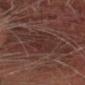Q: Is there a histopathology result?
A: total-body-photography surveillance lesion; no biopsy
Q: Automated lesion metrics?
A: a footprint of about 13 mm², a shape eccentricity near 0.65, and a symmetry-axis asymmetry near 0.3; an average lesion color of about L≈25 a*≈17 b*≈18 (CIELAB) and a normalized border contrast of about 5
Q: How was this image acquired?
A: ~15 mm tile from a whole-body skin photo
Q: What is the lesion's diameter?
A: about 5 mm
Q: Patient demographics?
A: male, aged 63 to 67
Q: Illumination type?
A: cross-polarized illumination
Q: Lesion location?
A: the head or neck